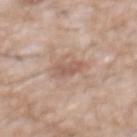Case summary:
– biopsy status · imaged on a skin check; not biopsied
– location · the chest
– tile lighting · white-light illumination
– size · ≈2.5 mm
– subject · male, aged approximately 60
– imaging modality · 15 mm crop, total-body photography